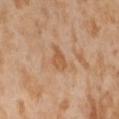workup=catalogued during a skin exam; not biopsied
lesion diameter=≈3 mm
location=the abdomen
image source=~15 mm tile from a whole-body skin photo
patient=female, aged approximately 55
illumination=cross-polarized